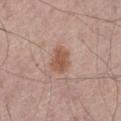Assessment: This lesion was catalogued during total-body skin photography and was not selected for biopsy. Context: A male patient aged around 55. Automated tile analysis of the lesion measured an average lesion color of about L≈54 a*≈21 b*≈29 (CIELAB), roughly 10 lightness units darker than nearby skin, and a lesion-to-skin contrast of about 7.5 (normalized; higher = more distinct). And it measured a classifier nevus-likeness of about 90/100 and lesion-presence confidence of about 100/100. Cropped from a total-body skin-imaging series; the visible field is about 15 mm. On the left thigh. The recorded lesion diameter is about 3 mm.